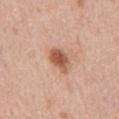No biopsy was performed on this lesion — it was imaged during a full skin examination and was not determined to be concerning. Located on the abdomen. An algorithmic analysis of the crop reported a mean CIELAB color near L≈56 a*≈23 b*≈32, a lesion–skin lightness drop of about 14, and a lesion-to-skin contrast of about 9 (normalized; higher = more distinct). The software also gave a border-irregularity index near 2.5/10, a color-variation rating of about 4.5/10, and peripheral color asymmetry of about 1.5. This is a white-light tile. A male subject, approximately 70 years of age. This image is a 15 mm lesion crop taken from a total-body photograph. Approximately 3.5 mm at its widest.